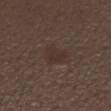| key | value |
|---|---|
| biopsy status | no biopsy performed (imaged during a skin exam) |
| image source | total-body-photography crop, ~15 mm field of view |
| tile lighting | white-light illumination |
| anatomic site | the arm |
| patient | female, about 50 years old |